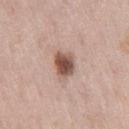Q: Was a biopsy performed?
A: no biopsy performed (imaged during a skin exam)
Q: What lighting was used for the tile?
A: white-light
Q: What did automated image analysis measure?
A: a mean CIELAB color near L≈52 a*≈20 b*≈25, a lesion–skin lightness drop of about 17, and a normalized lesion–skin contrast near 11; an automated nevus-likeness rating near 95 out of 100
Q: What is the imaging modality?
A: 15 mm crop, total-body photography
Q: What are the patient's age and sex?
A: female, aged around 40
Q: Where on the body is the lesion?
A: the right thigh